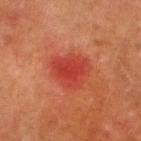Q: Is there a histopathology result?
A: imaged on a skin check; not biopsied
Q: Patient demographics?
A: male, in their 70s
Q: What is the imaging modality?
A: ~15 mm crop, total-body skin-cancer survey
Q: How was the tile lit?
A: cross-polarized
Q: What is the lesion's diameter?
A: ~4.5 mm (longest diameter)
Q: Where on the body is the lesion?
A: the right lower leg